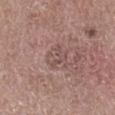• follow-up — catalogued during a skin exam; not biopsied
• patient — male, about 50 years old
• lesion size — ≈3 mm
• body site — the right lower leg
• imaging modality — ~15 mm crop, total-body skin-cancer survey